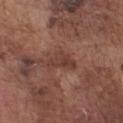Impression:
Imaged during a routine full-body skin examination; the lesion was not biopsied and no histopathology is available.
Context:
Captured under white-light illumination. An algorithmic analysis of the crop reported a lesion color around L≈39 a*≈22 b*≈25 in CIELAB and a lesion-to-skin contrast of about 6.5 (normalized; higher = more distinct). The analysis additionally found a border-irregularity rating of about 5/10, internal color variation of about 3 on a 0–10 scale, and peripheral color asymmetry of about 1. The subject is a male approximately 75 years of age. Longest diameter approximately 3.5 mm. A 15 mm close-up extracted from a 3D total-body photography capture. On the front of the torso.